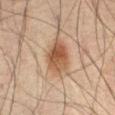A region of skin cropped from a whole-body photographic capture, roughly 15 mm wide. The lesion is located on the back. The subject is a male aged 68–72. About 4.5 mm across. The tile uses cross-polarized illumination. Automated tile analysis of the lesion measured a classifier nevus-likeness of about 95/100.